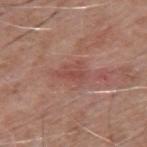<case>
<biopsy_status>not biopsied; imaged during a skin examination</biopsy_status>
<image>
  <source>total-body photography crop</source>
  <field_of_view_mm>15</field_of_view_mm>
</image>
<lighting>white-light</lighting>
<patient>
  <sex>male</sex>
  <age_approx>60</age_approx>
</patient>
<site>mid back</site>
</case>